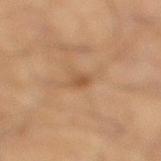Imaged during a routine full-body skin examination; the lesion was not biopsied and no histopathology is available. The lesion is located on the left lower leg. Cropped from a whole-body photographic skin survey; the tile spans about 15 mm. The tile uses cross-polarized illumination. An algorithmic analysis of the crop reported a lesion area of about 2.5 mm², a shape eccentricity near 0.9, and a shape-asymmetry score of about 0.4 (0 = symmetric). The recorded lesion diameter is about 3 mm. A male subject aged approximately 60.